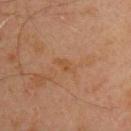{
  "biopsy_status": "not biopsied; imaged during a skin examination",
  "automated_metrics": {
    "cielab_L": 41,
    "cielab_a": 18,
    "cielab_b": 30,
    "vs_skin_contrast_norm": 5.0,
    "border_irregularity_0_10": 3.5,
    "color_variation_0_10": 1.0,
    "peripheral_color_asymmetry": 0.5,
    "lesion_detection_confidence_0_100": 100
  },
  "lesion_size": {
    "long_diameter_mm_approx": 2.5
  },
  "patient": {
    "sex": "male",
    "age_approx": 45
  },
  "lighting": "cross-polarized",
  "site": "front of the torso",
  "image": {
    "source": "total-body photography crop",
    "field_of_view_mm": 15
  }
}